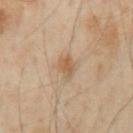Assessment:
Recorded during total-body skin imaging; not selected for excision or biopsy.
Acquisition and patient details:
A male subject, about 55 years old. Automated tile analysis of the lesion measured an area of roughly 4 mm² and a symmetry-axis asymmetry near 0.25. It also reported border irregularity of about 2.5 on a 0–10 scale, a color-variation rating of about 2/10, and peripheral color asymmetry of about 0.5. And it measured a nevus-likeness score of about 55/100. A close-up tile cropped from a whole-body skin photograph, about 15 mm across. The tile uses cross-polarized illumination. The lesion is located on the mid back. Longest diameter approximately 3 mm.